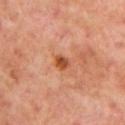Part of a total-body skin-imaging series; this lesion was reviewed on a skin check and was not flagged for biopsy.
Automated tile analysis of the lesion measured an area of roughly 3 mm², an outline eccentricity of about 0.55 (0 = round, 1 = elongated), and a shape-asymmetry score of about 0.3 (0 = symmetric).
Imaged with cross-polarized lighting.
The lesion is on the arm.
The patient is a male in their 50s.
Cropped from a total-body skin-imaging series; the visible field is about 15 mm.
Approximately 2 mm at its widest.